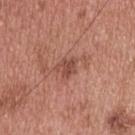image — ~15 mm crop, total-body skin-cancer survey
lesion size — ~2.5 mm (longest diameter)
patient — male, in their mid-50s
tile lighting — white-light
location — the upper back
TBP lesion metrics — a shape-asymmetry score of about 0.35 (0 = symmetric); a mean CIELAB color near L≈48 a*≈24 b*≈27 and about 9 CIELAB-L* units darker than the surrounding skin; a border-irregularity rating of about 3/10, a color-variation rating of about 2.5/10, and a peripheral color-asymmetry measure near 1